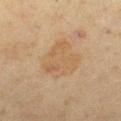Q: Was a biopsy performed?
A: total-body-photography surveillance lesion; no biopsy
Q: How large is the lesion?
A: about 5 mm
Q: What is the imaging modality?
A: total-body-photography crop, ~15 mm field of view
Q: What lighting was used for the tile?
A: cross-polarized illumination
Q: Automated lesion metrics?
A: a border-irregularity index near 2/10, a color-variation rating of about 2.5/10, and peripheral color asymmetry of about 1
Q: Lesion location?
A: the right lower leg
Q: Who is the patient?
A: female, aged 48–52